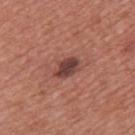notes: imaged on a skin check; not biopsied | acquisition: ~15 mm crop, total-body skin-cancer survey | subject: male, about 45 years old | lesion diameter: about 3.5 mm | anatomic site: the chest.